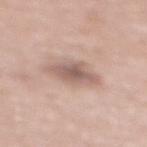<record>
  <biopsy_status>not biopsied; imaged during a skin examination</biopsy_status>
  <automated_metrics>
    <vs_skin_darker_L>11.0</vs_skin_darker_L>
    <vs_skin_contrast_norm>7.5</vs_skin_contrast_norm>
    <border_irregularity_0_10>2.5</border_irregularity_0_10>
    <color_variation_0_10>3.0</color_variation_0_10>
  </automated_metrics>
  <lesion_size>
    <long_diameter_mm_approx>4.5</long_diameter_mm_approx>
  </lesion_size>
  <lighting>white-light</lighting>
  <site>mid back</site>
  <image>
    <source>total-body photography crop</source>
    <field_of_view_mm>15</field_of_view_mm>
  </image>
  <patient>
    <sex>female</sex>
    <age_approx>70</age_approx>
  </patient>
</record>